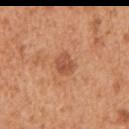Clinical impression: Part of a total-body skin-imaging series; this lesion was reviewed on a skin check and was not flagged for biopsy. Context: Located on the left upper arm. A male subject in their mid- to late 50s. Cropped from a total-body skin-imaging series; the visible field is about 15 mm.